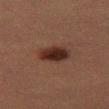Clinical impression: Part of a total-body skin-imaging series; this lesion was reviewed on a skin check and was not flagged for biopsy. Background: The lesion's longest dimension is about 4 mm. A 15 mm close-up tile from a total-body photography series done for melanoma screening. From the left thigh. This is a cross-polarized tile. A female patient, aged around 55. An algorithmic analysis of the crop reported a mean CIELAB color near L≈22 a*≈16 b*≈20, roughly 10 lightness units darker than nearby skin, and a normalized lesion–skin contrast near 11.5. The analysis additionally found border irregularity of about 2 on a 0–10 scale, internal color variation of about 3.5 on a 0–10 scale, and a peripheral color-asymmetry measure near 1.